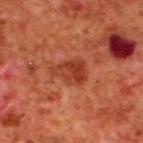Impression: Imaged during a routine full-body skin examination; the lesion was not biopsied and no histopathology is available. Background: Automated tile analysis of the lesion measured a lesion area of about 9 mm² and an eccentricity of roughly 0.75. The software also gave a mean CIELAB color near L≈40 a*≈32 b*≈36, about 9 CIELAB-L* units darker than the surrounding skin, and a normalized lesion–skin contrast near 7. A roughly 15 mm field-of-view crop from a total-body skin photograph. The lesion is on the upper back. The subject is a male aged around 70.